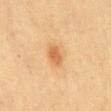{
  "biopsy_status": "not biopsied; imaged during a skin examination",
  "patient": {
    "sex": "female",
    "age_approx": 55
  },
  "lesion_size": {
    "long_diameter_mm_approx": 2.5
  },
  "automated_metrics": {
    "area_mm2_approx": 3.5,
    "shape_asymmetry": 0.25,
    "border_irregularity_0_10": 2.0,
    "color_variation_0_10": 1.5,
    "peripheral_color_asymmetry": 0.5
  },
  "image": {
    "source": "total-body photography crop",
    "field_of_view_mm": 15
  },
  "site": "mid back",
  "lighting": "cross-polarized"
}